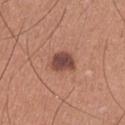Context:
The lesion is on the right upper arm. A male subject in their mid-30s. A close-up tile cropped from a whole-body skin photograph, about 15 mm across. Automated tile analysis of the lesion measured an eccentricity of roughly 0.6 and a symmetry-axis asymmetry near 0.25. The software also gave a mean CIELAB color near L≈45 a*≈23 b*≈25 and a normalized lesion–skin contrast near 10.5. It also reported border irregularity of about 2.5 on a 0–10 scale, a within-lesion color-variation index near 3/10, and radial color variation of about 1. The software also gave an automated nevus-likeness rating near 80 out of 100 and lesion-presence confidence of about 100/100.